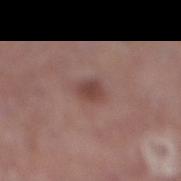The lesion was tiled from a total-body skin photograph and was not biopsied. The lesion-visualizer software estimated a lesion area of about 4 mm², an outline eccentricity of about 0.8 (0 = round, 1 = elongated), and two-axis asymmetry of about 0.25. The analysis additionally found an average lesion color of about L≈44 a*≈21 b*≈22 (CIELAB) and a normalized border contrast of about 7.5. This is a white-light tile. The subject is a female aged around 85 or older. A close-up tile cropped from a whole-body skin photograph, about 15 mm across. The lesion is on the right lower leg.